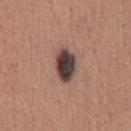{
  "biopsy_status": "not biopsied; imaged during a skin examination",
  "automated_metrics": {
    "border_irregularity_0_10": 2.0
  },
  "site": "chest",
  "lesion_size": {
    "long_diameter_mm_approx": 4.5
  },
  "lighting": "white-light",
  "image": {
    "source": "total-body photography crop",
    "field_of_view_mm": 15
  },
  "patient": {
    "sex": "male",
    "age_approx": 45
  }
}